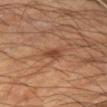No biopsy was performed on this lesion — it was imaged during a full skin examination and was not determined to be concerning. On the left forearm. Approximately 2.5 mm at its widest. Captured under cross-polarized illumination. The patient is a male roughly 55 years of age. This image is a 15 mm lesion crop taken from a total-body photograph.